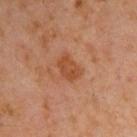Part of a total-body skin-imaging series; this lesion was reviewed on a skin check and was not flagged for biopsy.
A male patient, in their 60s.
Located on the right upper arm.
The tile uses cross-polarized illumination.
A region of skin cropped from a whole-body photographic capture, roughly 15 mm wide.
About 3.5 mm across.